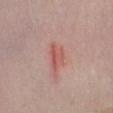follow-up = no biopsy performed (imaged during a skin exam)
subject = female, roughly 40 years of age
site = the left lower leg
automated metrics = a lesion area of about 5 mm²; border irregularity of about 4 on a 0–10 scale, a color-variation rating of about 4.5/10, and peripheral color asymmetry of about 1.5
acquisition = total-body-photography crop, ~15 mm field of view
lesion size = ~3.5 mm (longest diameter)